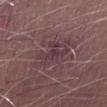Automated image analysis of the tile measured an area of roughly 8.5 mm² and two-axis asymmetry of about 0.3. It also reported an average lesion color of about L≈36 a*≈18 b*≈12 (CIELAB) and roughly 6 lightness units darker than nearby skin. The software also gave a classifier nevus-likeness of about 0/100 and a detector confidence of about 70 out of 100 that the crop contains a lesion. Measured at roughly 4 mm in maximum diameter. Located on the arm. Imaged with white-light lighting. A 15 mm close-up extracted from a 3D total-body photography capture. A male patient in their mid- to late 60s.